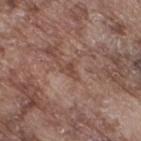The lesion was photographed on a routine skin check and not biopsied; there is no pathology result.
A 15 mm crop from a total-body photograph taken for skin-cancer surveillance.
The lesion-visualizer software estimated a lesion color around L≈45 a*≈19 b*≈25 in CIELAB and a normalized lesion–skin contrast near 6.5. And it measured a classifier nevus-likeness of about 0/100 and a detector confidence of about 70 out of 100 that the crop contains a lesion.
On the right thigh.
The tile uses white-light illumination.
The lesion's longest dimension is about 2.5 mm.
A male patient, aged approximately 75.